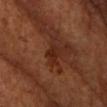This lesion was catalogued during total-body skin photography and was not selected for biopsy. A male patient, aged around 70. Located on the head or neck. About 3 mm across. An algorithmic analysis of the crop reported an average lesion color of about L≈25 a*≈23 b*≈28 (CIELAB), about 7 CIELAB-L* units darker than the surrounding skin, and a normalized lesion–skin contrast near 7. A roughly 15 mm field-of-view crop from a total-body skin photograph.